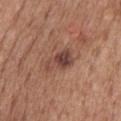{
  "biopsy_status": "not biopsied; imaged during a skin examination",
  "patient": {
    "sex": "male",
    "age_approx": 60
  },
  "site": "mid back",
  "automated_metrics": {
    "eccentricity": 0.85,
    "shape_asymmetry": 0.35,
    "border_irregularity_0_10": 3.5,
    "color_variation_0_10": 6.5,
    "peripheral_color_asymmetry": 2.0
  },
  "image": {
    "source": "total-body photography crop",
    "field_of_view_mm": 15
  },
  "lighting": "white-light",
  "lesion_size": {
    "long_diameter_mm_approx": 4.0
  }
}